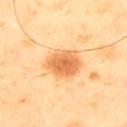anatomic site: the right upper arm
acquisition: total-body-photography crop, ~15 mm field of view
patient: male, approximately 45 years of age